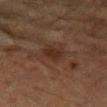Clinical impression: Imaged during a routine full-body skin examination; the lesion was not biopsied and no histopathology is available. Image and clinical context: A close-up tile cropped from a whole-body skin photograph, about 15 mm across. On the left forearm. The subject is a male aged 83 or older.